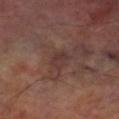Part of a total-body skin-imaging series; this lesion was reviewed on a skin check and was not flagged for biopsy. The tile uses cross-polarized illumination. A 15 mm close-up extracted from a 3D total-body photography capture. From the right lower leg. A male patient about 70 years old. Automated tile analysis of the lesion measured an area of roughly 4 mm². The analysis additionally found a lesion color around L≈33 a*≈18 b*≈19 in CIELAB, a lesion–skin lightness drop of about 5, and a normalized border contrast of about 5.5. And it measured internal color variation of about 2.5 on a 0–10 scale and peripheral color asymmetry of about 1. The software also gave an automated nevus-likeness rating near 0 out of 100. The recorded lesion diameter is about 3 mm.